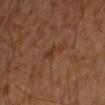Findings:
• biopsy status · total-body-photography surveillance lesion; no biopsy
• body site · the left forearm
• imaging modality · ~15 mm tile from a whole-body skin photo
• subject · male, aged 43 to 47
• tile lighting · cross-polarized illumination